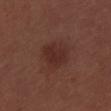Acquisition and patient details:
About 4 mm across. From the upper back. The tile uses white-light illumination. Cropped from a whole-body photographic skin survey; the tile spans about 15 mm. A male patient aged 28–32. Automated image analysis of the tile measured an average lesion color of about L≈29 a*≈21 b*≈23 (CIELAB) and a lesion–skin lightness drop of about 6. And it measured a border-irregularity index near 3/10, a color-variation rating of about 1.5/10, and a peripheral color-asymmetry measure near 0.5. And it measured a nevus-likeness score of about 75/100 and a lesion-detection confidence of about 100/100.